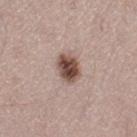Q: Was a biopsy performed?
A: catalogued during a skin exam; not biopsied
Q: Automated lesion metrics?
A: an outline eccentricity of about 0.65 (0 = round, 1 = elongated) and two-axis asymmetry of about 0.15; a mean CIELAB color near L≈49 a*≈18 b*≈24 and a lesion–skin lightness drop of about 17; a peripheral color-asymmetry measure near 2; a detector confidence of about 100 out of 100 that the crop contains a lesion
Q: What is the imaging modality?
A: total-body-photography crop, ~15 mm field of view
Q: Lesion location?
A: the leg
Q: How large is the lesion?
A: ~3.5 mm (longest diameter)
Q: Patient demographics?
A: female, in their 50s
Q: How was the tile lit?
A: white-light illumination